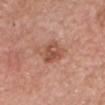Q: Who is the patient?
A: male, approximately 80 years of age
Q: What is the anatomic site?
A: the head or neck
Q: How large is the lesion?
A: ≈3.5 mm
Q: What is the imaging modality?
A: total-body-photography crop, ~15 mm field of view
Q: What did automated image analysis measure?
A: an area of roughly 6.5 mm², a shape eccentricity near 0.6, and two-axis asymmetry of about 0.25; a mean CIELAB color near L≈52 a*≈24 b*≈30, a lesion–skin lightness drop of about 10, and a lesion-to-skin contrast of about 7 (normalized; higher = more distinct); border irregularity of about 2.5 on a 0–10 scale and peripheral color asymmetry of about 1.5
Q: Illumination type?
A: white-light illumination